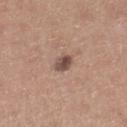The lesion was tiled from a total-body skin photograph and was not biopsied. This image is a 15 mm lesion crop taken from a total-body photograph. The lesion is on the right lower leg. The lesion-visualizer software estimated a within-lesion color-variation index near 4/10 and a peripheral color-asymmetry measure near 1.5. The lesion's longest dimension is about 2 mm. A male subject aged 58–62.